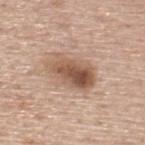<tbp_lesion>
<biopsy_status>not biopsied; imaged during a skin examination</biopsy_status>
</tbp_lesion>